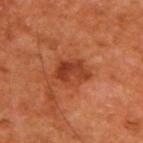workup: no biopsy performed (imaged during a skin exam) | body site: the upper back | image: total-body-photography crop, ~15 mm field of view | subject: male, aged around 65.